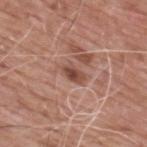No biopsy was performed on this lesion — it was imaged during a full skin examination and was not determined to be concerning.
A male subject aged around 60.
Automated image analysis of the tile measured a footprint of about 3.5 mm², an eccentricity of roughly 0.8, and a symmetry-axis asymmetry near 0.2. And it measured roughly 12 lightness units darker than nearby skin and a lesion-to-skin contrast of about 8.5 (normalized; higher = more distinct).
A 15 mm close-up tile from a total-body photography series done for melanoma screening.
Located on the upper back.
Imaged with white-light lighting.
Measured at roughly 2.5 mm in maximum diameter.